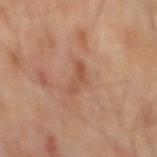| feature | finding |
|---|---|
| patient | male, in their mid- to late 60s |
| image-analysis metrics | a footprint of about 4 mm², a shape eccentricity near 0.9, and a symmetry-axis asymmetry near 0.5; border irregularity of about 6 on a 0–10 scale and radial color variation of about 0 |
| lesion size | ~3.5 mm (longest diameter) |
| image | ~15 mm tile from a whole-body skin photo |
| tile lighting | cross-polarized illumination |
| anatomic site | the mid back |